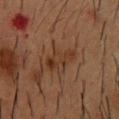| feature | finding |
|---|---|
| follow-up | imaged on a skin check; not biopsied |
| patient | male, in their mid- to late 50s |
| acquisition | total-body-photography crop, ~15 mm field of view |
| lighting | cross-polarized illumination |
| automated metrics | a shape eccentricity near 0.85 and two-axis asymmetry of about 0.3; border irregularity of about 4 on a 0–10 scale and a within-lesion color-variation index near 4.5/10; a detector confidence of about 100 out of 100 that the crop contains a lesion |
| location | the chest |
| size | ~4 mm (longest diameter) |